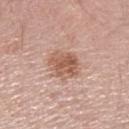- notes: catalogued during a skin exam; not biopsied
- patient: female, about 55 years old
- site: the left forearm
- imaging modality: ~15 mm tile from a whole-body skin photo
- lighting: white-light
- lesion size: ≈4 mm